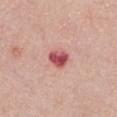Notes:
- notes — total-body-photography surveillance lesion; no biopsy
- patient — female, aged around 55
- anatomic site — the chest
- acquisition — ~15 mm crop, total-body skin-cancer survey
- lighting — white-light illumination